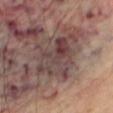Background:
This image is a 15 mm lesion crop taken from a total-body photograph. The subject is a male roughly 70 years of age. Longest diameter approximately 6.5 mm. On the right thigh. Imaged with cross-polarized lighting.